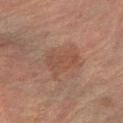Case summary:
- workup: imaged on a skin check; not biopsied
- image: ~15 mm crop, total-body skin-cancer survey
- size: ~4 mm (longest diameter)
- lighting: cross-polarized illumination
- site: the right forearm
- patient: female, roughly 70 years of age